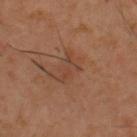Q: Is there a histopathology result?
A: total-body-photography surveillance lesion; no biopsy
Q: Where on the body is the lesion?
A: the upper back
Q: Automated lesion metrics?
A: a border-irregularity rating of about 8/10, a within-lesion color-variation index near 0/10, and peripheral color asymmetry of about 0
Q: How was this image acquired?
A: total-body-photography crop, ~15 mm field of view
Q: How large is the lesion?
A: ≈3.5 mm
Q: What lighting was used for the tile?
A: cross-polarized illumination
Q: Who is the patient?
A: male, in their 40s